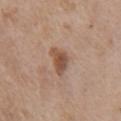biopsy_status: not biopsied; imaged during a skin examination
site: chest
patient:
  sex: female
  age_approx: 75
automated_metrics:
  cielab_L: 51
  cielab_a: 19
  cielab_b: 29
  vs_skin_darker_L: 11.0
  vs_skin_contrast_norm: 8.5
  color_variation_0_10: 4.0
  peripheral_color_asymmetry: 1.5
  nevus_likeness_0_100: 70
  lesion_detection_confidence_0_100: 100
image:
  source: total-body photography crop
  field_of_view_mm: 15
lighting: white-light
lesion_size:
  long_diameter_mm_approx: 3.0